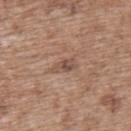{"biopsy_status": "not biopsied; imaged during a skin examination", "image": {"source": "total-body photography crop", "field_of_view_mm": 15}, "patient": {"sex": "male", "age_approx": 70}, "site": "back"}